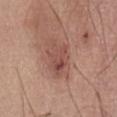Q: Was this lesion biopsied?
A: total-body-photography surveillance lesion; no biopsy
Q: Lesion size?
A: ~5 mm (longest diameter)
Q: What kind of image is this?
A: ~15 mm crop, total-body skin-cancer survey
Q: Lesion location?
A: the front of the torso
Q: Who is the patient?
A: male, about 60 years old